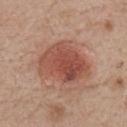Case summary:
- workup: no biopsy performed (imaged during a skin exam)
- anatomic site: the chest
- subject: male, in their mid-70s
- image-analysis metrics: a border-irregularity index near 2.5/10, a color-variation rating of about 5.5/10, and peripheral color asymmetry of about 2
- tile lighting: white-light
- image source: ~15 mm crop, total-body skin-cancer survey